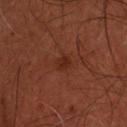biopsy_status: not biopsied; imaged during a skin examination
site: upper back
lesion_size:
  long_diameter_mm_approx: 2.0
image:
  source: total-body photography crop
  field_of_view_mm: 15
lighting: cross-polarized
patient:
  sex: male
  age_approx: 45
automated_metrics:
  area_mm2_approx: 3.0
  eccentricity: 0.25
  border_irregularity_0_10: 2.0
  nevus_likeness_0_100: 0